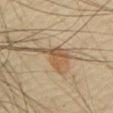The lesion was photographed on a routine skin check and not biopsied; there is no pathology result.
The lesion is located on the chest.
Imaged with cross-polarized lighting.
The patient is a male about 65 years old.
A roughly 15 mm field-of-view crop from a total-body skin photograph.